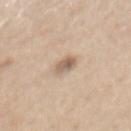Assessment: The lesion was tiled from a total-body skin photograph and was not biopsied. Acquisition and patient details: Cropped from a whole-body photographic skin survey; the tile spans about 15 mm. On the mid back. The patient is a female aged 43–47. An algorithmic analysis of the crop reported a border-irregularity index near 2/10, internal color variation of about 4 on a 0–10 scale, and peripheral color asymmetry of about 1.5. And it measured a nevus-likeness score of about 50/100 and a lesion-detection confidence of about 100/100.